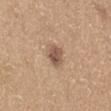Impression: The lesion was photographed on a routine skin check and not biopsied; there is no pathology result. Background: On the lower back. The recorded lesion diameter is about 3 mm. A 15 mm close-up tile from a total-body photography series done for melanoma screening. The subject is a male in their mid-60s. The tile uses white-light illumination.